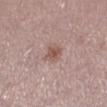biopsy status — catalogued during a skin exam; not biopsied
size — ≈2.5 mm
tile lighting — white-light
site — the right lower leg
subject — female, in their 50s
imaging modality — ~15 mm crop, total-body skin-cancer survey
automated lesion analysis — a footprint of about 5.5 mm², an outline eccentricity of about 0.4 (0 = round, 1 = elongated), and a symmetry-axis asymmetry near 0.3; a mean CIELAB color near L≈54 a*≈19 b*≈23 and a lesion-to-skin contrast of about 6.5 (normalized; higher = more distinct); border irregularity of about 3 on a 0–10 scale and internal color variation of about 3 on a 0–10 scale